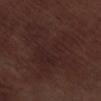Q: Is there a histopathology result?
A: total-body-photography surveillance lesion; no biopsy
Q: Automated lesion metrics?
A: border irregularity of about 8 on a 0–10 scale and a color-variation rating of about 2.5/10
Q: What lighting was used for the tile?
A: white-light
Q: What is the lesion's diameter?
A: ~9.5 mm (longest diameter)
Q: What kind of image is this?
A: ~15 mm tile from a whole-body skin photo
Q: What are the patient's age and sex?
A: male, in their 70s
Q: Where on the body is the lesion?
A: the right lower leg